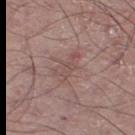Captured during whole-body skin photography for melanoma surveillance; the lesion was not biopsied. A male patient aged approximately 55. A lesion tile, about 15 mm wide, cut from a 3D total-body photograph. Located on the right thigh. Automated image analysis of the tile measured a footprint of about 6.5 mm², a shape eccentricity near 0.85, and two-axis asymmetry of about 0.4. The software also gave an average lesion color of about L≈50 a*≈18 b*≈20 (CIELAB), about 6 CIELAB-L* units darker than the surrounding skin, and a normalized border contrast of about 4.5. The software also gave border irregularity of about 5 on a 0–10 scale, a within-lesion color-variation index near 3/10, and a peripheral color-asymmetry measure near 1. The analysis additionally found a nevus-likeness score of about 0/100. Imaged with white-light lighting.